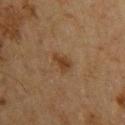follow-up = total-body-photography surveillance lesion; no biopsy | size = about 2.5 mm | image = ~15 mm tile from a whole-body skin photo | automated lesion analysis = an average lesion color of about L≈34 a*≈17 b*≈30 (CIELAB), roughly 8 lightness units darker than nearby skin, and a normalized lesion–skin contrast near 8; border irregularity of about 3.5 on a 0–10 scale, internal color variation of about 1.5 on a 0–10 scale, and a peripheral color-asymmetry measure near 0.5; a lesion-detection confidence of about 100/100 | patient = male, approximately 60 years of age | tile lighting = cross-polarized.